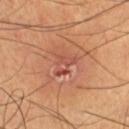Part of a total-body skin-imaging series; this lesion was reviewed on a skin check and was not flagged for biopsy.
Located on the left lower leg.
The lesion-visualizer software estimated a shape-asymmetry score of about 0.35 (0 = symmetric). The analysis additionally found internal color variation of about 4 on a 0–10 scale. It also reported an automated nevus-likeness rating near 0 out of 100 and a lesion-detection confidence of about 95/100.
Cropped from a total-body skin-imaging series; the visible field is about 15 mm.
Measured at roughly 3 mm in maximum diameter.
This is a cross-polarized tile.
A male patient aged 38 to 42.